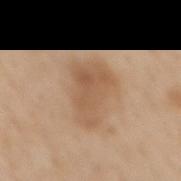  lesion_size:
    long_diameter_mm_approx: 5.5
  image:
    source: total-body photography crop
    field_of_view_mm: 15
  automated_metrics:
    area_mm2_approx: 16.0
    eccentricity: 0.8
    cielab_L: 57
    cielab_a: 18
    cielab_b: 33
    vs_skin_darker_L: 9.0
    color_variation_0_10: 3.0
    nevus_likeness_0_100: 10
    lesion_detection_confidence_0_100: 100
  patient:
    sex: female
    age_approx: 65
  site: mid back